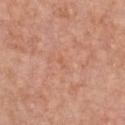notes: no biopsy performed (imaged during a skin exam) | automated metrics: an area of roughly 1 mm², an eccentricity of roughly 0.85, and a symmetry-axis asymmetry near 0.45; a border-irregularity rating of about 3.5/10, a within-lesion color-variation index near 0/10, and a peripheral color-asymmetry measure near 0 | acquisition: total-body-photography crop, ~15 mm field of view | body site: the chest | subject: female, in their mid-40s | diameter: ~1.5 mm (longest diameter).